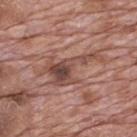| field | value |
|---|---|
| follow-up | total-body-photography surveillance lesion; no biopsy |
| site | the mid back |
| subject | male, approximately 70 years of age |
| tile lighting | white-light |
| image | 15 mm crop, total-body photography |
| size | ~7 mm (longest diameter) |
| automated metrics | a footprint of about 12 mm², a shape eccentricity near 0.9, and two-axis asymmetry of about 0.7; roughly 11 lightness units darker than nearby skin and a normalized border contrast of about 8; border irregularity of about 10 on a 0–10 scale, a within-lesion color-variation index near 6.5/10, and a peripheral color-asymmetry measure near 2 |